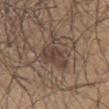This lesion was catalogued during total-body skin photography and was not selected for biopsy. On the chest. This is a white-light tile. The lesion-visualizer software estimated border irregularity of about 6.5 on a 0–10 scale, a within-lesion color-variation index near 2.5/10, and radial color variation of about 1. This image is a 15 mm lesion crop taken from a total-body photograph. A male patient aged around 40. The lesion's longest dimension is about 6.5 mm.